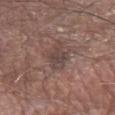Impression: The lesion was photographed on a routine skin check and not biopsied; there is no pathology result. Context: Automated tile analysis of the lesion measured radial color variation of about 0.5. From the left forearm. A male patient aged 78–82. The recorded lesion diameter is about 4 mm. A close-up tile cropped from a whole-body skin photograph, about 15 mm across. This is a white-light tile.